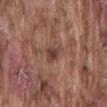Q: Is there a histopathology result?
A: total-body-photography surveillance lesion; no biopsy
Q: What is the lesion's diameter?
A: ~3.5 mm (longest diameter)
Q: Where on the body is the lesion?
A: the back
Q: What kind of image is this?
A: ~15 mm tile from a whole-body skin photo
Q: Who is the patient?
A: male, roughly 75 years of age